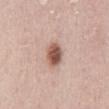Recorded during total-body skin imaging; not selected for excision or biopsy.
Measured at roughly 3.5 mm in maximum diameter.
On the abdomen.
The subject is a female aged 28–32.
Imaged with white-light lighting.
A close-up tile cropped from a whole-body skin photograph, about 15 mm across.